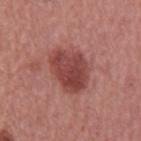biopsy status: total-body-photography surveillance lesion; no biopsy | acquisition: 15 mm crop, total-body photography | site: the mid back | patient: male, aged 63–67.